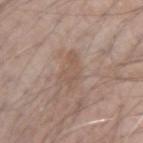No biopsy was performed on this lesion — it was imaged during a full skin examination and was not determined to be concerning. About 4.5 mm across. A 15 mm close-up tile from a total-body photography series done for melanoma screening. A male subject, aged 68 to 72. The lesion is located on the left forearm. The tile uses white-light illumination.